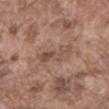This lesion was catalogued during total-body skin photography and was not selected for biopsy. From the abdomen. Longest diameter approximately 4.5 mm. The subject is a male about 75 years old. A 15 mm crop from a total-body photograph taken for skin-cancer surveillance.